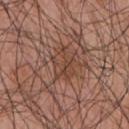Notes:
- workup · imaged on a skin check; not biopsied
- lesion diameter · ≈4 mm
- illumination · white-light
- subject · male, in their mid- to late 50s
- acquisition · 15 mm crop, total-body photography
- anatomic site · the front of the torso